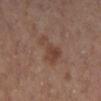<case>
<biopsy_status>not biopsied; imaged during a skin examination</biopsy_status>
<automated_metrics>
  <cielab_L>43</cielab_L>
  <cielab_a>19</cielab_a>
  <cielab_b>26</cielab_b>
  <vs_skin_darker_L>7.0</vs_skin_darker_L>
  <vs_skin_contrast_norm>6.0</vs_skin_contrast_norm>
  <border_irregularity_0_10>5.5</border_irregularity_0_10>
  <color_variation_0_10>4.0</color_variation_0_10>
  <peripheral_color_asymmetry>1.5</peripheral_color_asymmetry>
  <nevus_likeness_0_100>0</nevus_likeness_0_100>
</automated_metrics>
<patient>
  <sex>female</sex>
  <age_approx>40</age_approx>
</patient>
<image>
  <source>total-body photography crop</source>
  <field_of_view_mm>15</field_of_view_mm>
</image>
<site>right lower leg</site>
<lesion_size>
  <long_diameter_mm_approx>5.0</long_diameter_mm_approx>
</lesion_size>
<lighting>white-light</lighting>
</case>